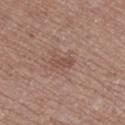{
  "biopsy_status": "not biopsied; imaged during a skin examination",
  "automated_metrics": {
    "color_variation_0_10": 0.0,
    "peripheral_color_asymmetry": 0.0,
    "nevus_likeness_0_100": 0,
    "lesion_detection_confidence_0_100": 100
  },
  "lesion_size": {
    "long_diameter_mm_approx": 2.5
  },
  "lighting": "white-light",
  "site": "right thigh",
  "image": {
    "source": "total-body photography crop",
    "field_of_view_mm": 15
  },
  "patient": {
    "sex": "male",
    "age_approx": 65
  }
}